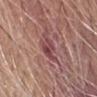Imaged during a routine full-body skin examination; the lesion was not biopsied and no histopathology is available.
A male subject, aged approximately 65.
Imaged with white-light lighting.
The recorded lesion diameter is about 3.5 mm.
On the right forearm.
This image is a 15 mm lesion crop taken from a total-body photograph.
Automated tile analysis of the lesion measured a lesion area of about 6 mm² and an outline eccentricity of about 0.75 (0 = round, 1 = elongated). It also reported an average lesion color of about L≈45 a*≈26 b*≈19 (CIELAB) and a normalized border contrast of about 7. And it measured border irregularity of about 4.5 on a 0–10 scale, internal color variation of about 4.5 on a 0–10 scale, and radial color variation of about 2. The analysis additionally found a lesion-detection confidence of about 95/100.